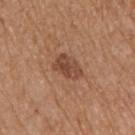Findings:
- workup: total-body-photography surveillance lesion; no biopsy
- site: the left upper arm
- lighting: white-light
- patient: male, roughly 70 years of age
- lesion diameter: about 3.5 mm
- image: total-body-photography crop, ~15 mm field of view